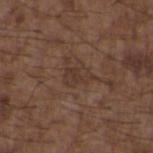Assessment: Recorded during total-body skin imaging; not selected for excision or biopsy. Background: A male subject approximately 50 years of age. A 15 mm close-up tile from a total-body photography series done for melanoma screening. From the upper back.